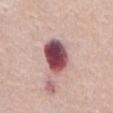| feature | finding |
|---|---|
| workup | imaged on a skin check; not biopsied |
| image source | ~15 mm crop, total-body skin-cancer survey |
| location | the abdomen |
| automated lesion analysis | a lesion color around L≈48 a*≈27 b*≈16 in CIELAB, roughly 24 lightness units darker than nearby skin, and a lesion-to-skin contrast of about 15.5 (normalized; higher = more distinct) |
| size | ≈4.5 mm |
| subject | female, about 70 years old |
| tile lighting | white-light |